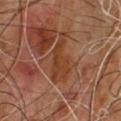This lesion was catalogued during total-body skin photography and was not selected for biopsy. Imaged with cross-polarized lighting. The patient is a male in their mid- to late 60s. A close-up tile cropped from a whole-body skin photograph, about 15 mm across. An algorithmic analysis of the crop reported an automated nevus-likeness rating near 0 out of 100 and a lesion-detection confidence of about 70/100.